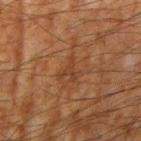Captured during whole-body skin photography for melanoma surveillance; the lesion was not biopsied. The lesion's longest dimension is about 3 mm. The lesion is on the arm. The lesion-visualizer software estimated border irregularity of about 8 on a 0–10 scale, a color-variation rating of about 0/10, and peripheral color asymmetry of about 0. It also reported a classifier nevus-likeness of about 0/100 and a detector confidence of about 95 out of 100 that the crop contains a lesion. Cropped from a whole-body photographic skin survey; the tile spans about 15 mm. A male subject aged approximately 65.